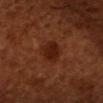Findings:
- workup · catalogued during a skin exam; not biopsied
- patient · male, aged around 50
- site · the head or neck
- acquisition · ~15 mm tile from a whole-body skin photo
- lighting · cross-polarized